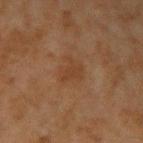Imaged during a routine full-body skin examination; the lesion was not biopsied and no histopathology is available. Imaged with cross-polarized lighting. A male patient aged 43–47. The lesion is on the right upper arm. Measured at roughly 3 mm in maximum diameter. A 15 mm crop from a total-body photograph taken for skin-cancer surveillance. Automated image analysis of the tile measured a footprint of about 5 mm². And it measured an automated nevus-likeness rating near 0 out of 100 and lesion-presence confidence of about 100/100.